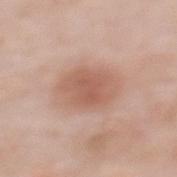site=the upper back | illumination=white-light | patient=female, approximately 50 years of age | image source=total-body-photography crop, ~15 mm field of view | lesion diameter=≈5.5 mm.